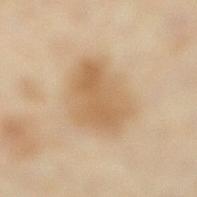Q: Was a biopsy performed?
A: catalogued during a skin exam; not biopsied
Q: Lesion location?
A: the right lower leg
Q: What lighting was used for the tile?
A: cross-polarized illumination
Q: Who is the patient?
A: female, in their mid-30s
Q: What kind of image is this?
A: total-body-photography crop, ~15 mm field of view
Q: What is the lesion's diameter?
A: about 6.5 mm